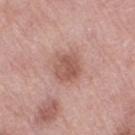| field | value |
|---|---|
| biopsy status | total-body-photography surveillance lesion; no biopsy |
| automated lesion analysis | a shape eccentricity near 0.4 and two-axis asymmetry of about 0.2 |
| image source | ~15 mm crop, total-body skin-cancer survey |
| anatomic site | the right thigh |
| lesion size | ~3.5 mm (longest diameter) |
| patient | female, about 70 years old |
| lighting | white-light |